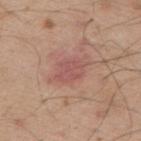site = the upper back; acquisition = ~15 mm crop, total-body skin-cancer survey; subject = male, roughly 55 years of age.